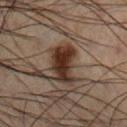Clinical impression: No biopsy was performed on this lesion — it was imaged during a full skin examination and was not determined to be concerning. Clinical summary: Imaged with cross-polarized lighting. The lesion is located on the right lower leg. The total-body-photography lesion software estimated a lesion area of about 10 mm² and a symmetry-axis asymmetry near 0.35. The analysis additionally found a peripheral color-asymmetry measure near 1. The lesion's longest dimension is about 4 mm. Cropped from a whole-body photographic skin survey; the tile spans about 15 mm. The patient is a male in their 50s.